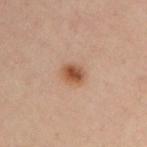Q: Was a biopsy performed?
A: catalogued during a skin exam; not biopsied
Q: How was this image acquired?
A: total-body-photography crop, ~15 mm field of view
Q: Patient demographics?
A: male, approximately 35 years of age
Q: Lesion location?
A: the left arm